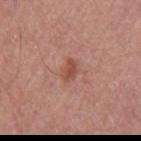workup: total-body-photography surveillance lesion; no biopsy
image: total-body-photography crop, ~15 mm field of view
lesion diameter: ~2.5 mm (longest diameter)
body site: the left upper arm
subject: male, in their mid- to late 60s